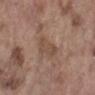* follow-up — imaged on a skin check; not biopsied